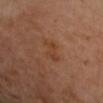Clinical summary: Cropped from a whole-body photographic skin survey; the tile spans about 15 mm. This is a cross-polarized tile. A female subject, approximately 55 years of age. The lesion is on the arm. Automated image analysis of the tile measured border irregularity of about 3.5 on a 0–10 scale, a within-lesion color-variation index near 2/10, and a peripheral color-asymmetry measure near 0.5. The software also gave a classifier nevus-likeness of about 0/100 and a lesion-detection confidence of about 100/100.